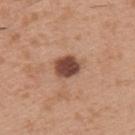workup = no biopsy performed (imaged during a skin exam) | imaging modality = 15 mm crop, total-body photography | subject = male, aged approximately 30 | diameter = about 3 mm | illumination = white-light illumination | body site = the upper back.